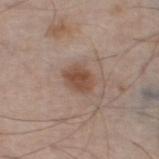The lesion was tiled from a total-body skin photograph and was not biopsied. A male subject in their 60s. Approximately 3 mm at its widest. The tile uses white-light illumination. Located on the left thigh. A 15 mm close-up extracted from a 3D total-body photography capture.